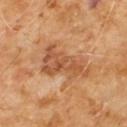biopsy status = no biopsy performed (imaged during a skin exam); location = the chest; diameter = about 6.5 mm; TBP lesion metrics = a mean CIELAB color near L≈51 a*≈23 b*≈36 and roughly 10 lightness units darker than nearby skin; lighting = cross-polarized; image = ~15 mm crop, total-body skin-cancer survey; patient = male, about 60 years old.